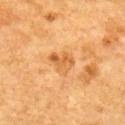Assessment:
Imaged during a routine full-body skin examination; the lesion was not biopsied and no histopathology is available.
Clinical summary:
A male subject aged 58–62. Cropped from a total-body skin-imaging series; the visible field is about 15 mm. Approximately 3 mm at its widest. From the upper back.